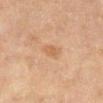Q: Was this lesion biopsied?
A: catalogued during a skin exam; not biopsied
Q: Lesion size?
A: ≈3 mm
Q: Patient demographics?
A: female, aged around 55
Q: Lesion location?
A: the right lower leg
Q: What kind of image is this?
A: total-body-photography crop, ~15 mm field of view